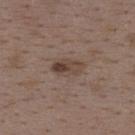Assessment: The lesion was photographed on a routine skin check and not biopsied; there is no pathology result. Clinical summary: On the upper back. Cropped from a whole-body photographic skin survey; the tile spans about 15 mm. A female patient approximately 35 years of age.